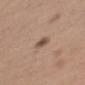This lesion was catalogued during total-body skin photography and was not selected for biopsy.
This is a white-light tile.
The lesion is located on the arm.
About 2.5 mm across.
The patient is a male aged 53 to 57.
The lesion-visualizer software estimated a lesion area of about 3.5 mm², an eccentricity of roughly 0.8, and a symmetry-axis asymmetry near 0.25. The analysis additionally found a lesion–skin lightness drop of about 11 and a normalized lesion–skin contrast near 8. The software also gave a nevus-likeness score of about 95/100.
A roughly 15 mm field-of-view crop from a total-body skin photograph.